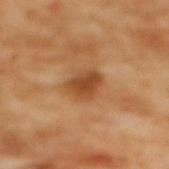No biopsy was performed on this lesion — it was imaged during a full skin examination and was not determined to be concerning.